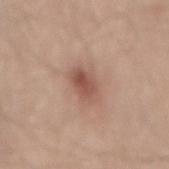Q: Is there a histopathology result?
A: imaged on a skin check; not biopsied
Q: Where on the body is the lesion?
A: the mid back
Q: What is the lesion's diameter?
A: ~4 mm (longest diameter)
Q: How was the tile lit?
A: white-light illumination
Q: What are the patient's age and sex?
A: male, aged around 45
Q: What is the imaging modality?
A: total-body-photography crop, ~15 mm field of view